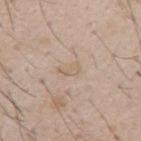Imaged during a routine full-body skin examination; the lesion was not biopsied and no histopathology is available. Imaged with white-light lighting. Automated image analysis of the tile measured an area of roughly 3 mm², an eccentricity of roughly 0.85, and a symmetry-axis asymmetry near 0.5. It also reported an average lesion color of about L≈62 a*≈13 b*≈29 (CIELAB) and about 6 CIELAB-L* units darker than the surrounding skin. A male subject, in their mid- to late 50s. About 2.5 mm across. A close-up tile cropped from a whole-body skin photograph, about 15 mm across.